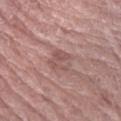Impression:
Captured during whole-body skin photography for melanoma surveillance; the lesion was not biopsied.
Image and clinical context:
The tile uses white-light illumination. This image is a 15 mm lesion crop taken from a total-body photograph. A male patient, aged approximately 65. The total-body-photography lesion software estimated a lesion area of about 6 mm² and a shape eccentricity near 0.35. Located on the arm.